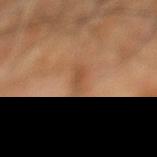Notes:
– workup · imaged on a skin check; not biopsied
– diameter · about 3 mm
– TBP lesion metrics · a lesion area of about 2.5 mm² and an eccentricity of roughly 0.95
– location · the leg
– subject · male, aged 68 to 72
– imaging modality · ~15 mm tile from a whole-body skin photo